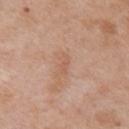Background: Approximately 2.5 mm at its widest. The tile uses white-light illumination. A roughly 15 mm field-of-view crop from a total-body skin photograph. The patient is a female aged 38 to 42. Located on the right upper arm.